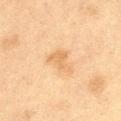Findings:
- workup — catalogued during a skin exam; not biopsied
- image source — ~15 mm crop, total-body skin-cancer survey
- illumination — cross-polarized
- subject — male, aged 53–57
- anatomic site — the leg